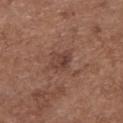biopsy_status: not biopsied; imaged during a skin examination
patient:
  sex: female
  age_approx: 75
image:
  source: total-body photography crop
  field_of_view_mm: 15
lighting: white-light
automated_metrics:
  area_mm2_approx: 4.0
  eccentricity: 0.6
  shape_asymmetry: 0.25
  cielab_L: 40
  cielab_a: 20
  cielab_b: 24
  vs_skin_contrast_norm: 6.5
  nevus_likeness_0_100: 0
  lesion_detection_confidence_0_100: 100
lesion_size:
  long_diameter_mm_approx: 2.5
site: back